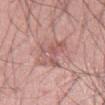follow-up: total-body-photography surveillance lesion; no biopsy
tile lighting: white-light
subject: male, in their mid- to late 40s
body site: the left thigh
lesion diameter: ≈5.5 mm
image: ~15 mm tile from a whole-body skin photo